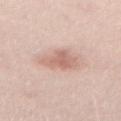patient: male, aged approximately 50
TBP lesion metrics: an automated nevus-likeness rating near 40 out of 100 and a lesion-detection confidence of about 100/100
tile lighting: white-light
diameter: about 3.5 mm
imaging modality: total-body-photography crop, ~15 mm field of view
body site: the right thigh